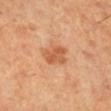workup: no biopsy performed (imaged during a skin exam) | patient: female, in their 50s | image source: ~15 mm tile from a whole-body skin photo.